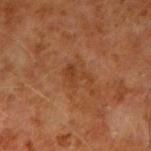| feature | finding |
|---|---|
| biopsy status | no biopsy performed (imaged during a skin exam) |
| acquisition | total-body-photography crop, ~15 mm field of view |
| body site | the arm |
| subject | male, aged 58–62 |